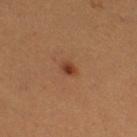Imaged during a routine full-body skin examination; the lesion was not biopsied and no histopathology is available.